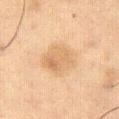notes: catalogued during a skin exam; not biopsied | subject: male, in their mid-50s | tile lighting: cross-polarized | body site: the right thigh | lesion size: about 5 mm | image: ~15 mm tile from a whole-body skin photo | automated lesion analysis: a lesion color around L≈62 a*≈16 b*≈35 in CIELAB, a lesion–skin lightness drop of about 7, and a normalized lesion–skin contrast near 5.5.